From the back. The subject is a male roughly 50 years of age. Cropped from a total-body skin-imaging series; the visible field is about 15 mm.
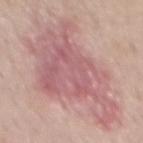The total-body-photography lesion software estimated a lesion color around L≈60 a*≈24 b*≈20 in CIELAB, about 10 CIELAB-L* units darker than the surrounding skin, and a normalized border contrast of about 7. The software also gave a border-irregularity index near 8/10 and peripheral color asymmetry of about 1.5. The software also gave an automated nevus-likeness rating near 0 out of 100 and a detector confidence of about 100 out of 100 that the crop contains a lesion.
The recorded lesion diameter is about 13 mm.
On biopsy, histopathology showed a superficial basal cell carcinoma.